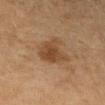• follow-up: catalogued during a skin exam; not biopsied
• body site: the left forearm
• imaging modality: 15 mm crop, total-body photography
• patient: female, aged 38 to 42